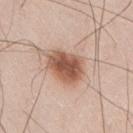Findings:
- biopsy status: total-body-photography surveillance lesion; no biopsy
- patient: male, aged around 60
- body site: the right thigh
- image: 15 mm crop, total-body photography
- lesion diameter: ~4.5 mm (longest diameter)
- tile lighting: white-light